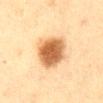Recorded during total-body skin imaging; not selected for excision or biopsy. A female patient, aged approximately 55. A region of skin cropped from a whole-body photographic capture, roughly 15 mm wide. The lesion is located on the front of the torso.